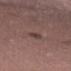Assessment: Part of a total-body skin-imaging series; this lesion was reviewed on a skin check and was not flagged for biopsy.